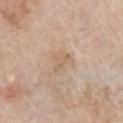Impression:
Captured during whole-body skin photography for melanoma surveillance; the lesion was not biopsied.
Background:
An algorithmic analysis of the crop reported a lesion area of about 5.5 mm² and an eccentricity of roughly 0.6. The analysis additionally found an average lesion color of about L≈63 a*≈15 b*≈31 (CIELAB), about 6 CIELAB-L* units darker than the surrounding skin, and a normalized lesion–skin contrast near 4.5. The analysis additionally found a border-irregularity rating of about 4.5/10, a color-variation rating of about 2/10, and peripheral color asymmetry of about 0.5. About 3 mm across. A roughly 15 mm field-of-view crop from a total-body skin photograph. Captured under white-light illumination. The subject is a male aged around 60. The lesion is located on the chest.